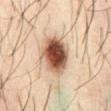patient: male, in their mid-40s
acquisition: total-body-photography crop, ~15 mm field of view
size: about 5 mm
lighting: cross-polarized illumination
image-analysis metrics: a footprint of about 15 mm², a shape eccentricity near 0.65, and two-axis asymmetry of about 0.15; an automated nevus-likeness rating near 100 out of 100 and a detector confidence of about 100 out of 100 that the crop contains a lesion
anatomic site: the front of the torso
histopathology: a dysplastic (Clark) nevus